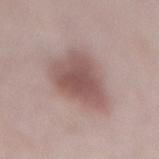Notes:
- biopsy status — total-body-photography surveillance lesion; no biopsy
- subject — male, aged 53 to 57
- anatomic site — the mid back
- lesion size — ≈5.5 mm
- acquisition — total-body-photography crop, ~15 mm field of view
- tile lighting — white-light illumination